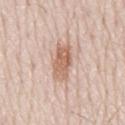This lesion was catalogued during total-body skin photography and was not selected for biopsy. Captured under white-light illumination. The lesion is located on the mid back. Cropped from a total-body skin-imaging series; the visible field is about 15 mm. A male patient, aged approximately 80. The total-body-photography lesion software estimated an average lesion color of about L≈63 a*≈19 b*≈29 (CIELAB) and a normalized lesion–skin contrast near 8. And it measured border irregularity of about 2.5 on a 0–10 scale, a color-variation rating of about 4/10, and peripheral color asymmetry of about 1.5.